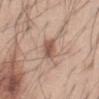Assessment:
No biopsy was performed on this lesion — it was imaged during a full skin examination and was not determined to be concerning.
Clinical summary:
From the abdomen. A male subject aged approximately 45. Measured at roughly 3 mm in maximum diameter. This is a white-light tile. A close-up tile cropped from a whole-body skin photograph, about 15 mm across.